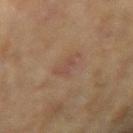Clinical impression: This lesion was catalogued during total-body skin photography and was not selected for biopsy. Background: This is a cross-polarized tile. A female patient aged around 80. A lesion tile, about 15 mm wide, cut from a 3D total-body photograph. From the right forearm.